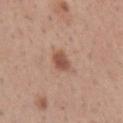Recorded during total-body skin imaging; not selected for excision or biopsy. A roughly 15 mm field-of-view crop from a total-body skin photograph. A female patient, in their 30s. The lesion is on the left forearm.